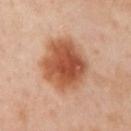Impression:
Recorded during total-body skin imaging; not selected for excision or biopsy.
Clinical summary:
The lesion is on the left arm. Cropped from a total-body skin-imaging series; the visible field is about 15 mm. A female patient, in their 40s.